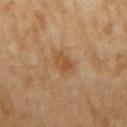Findings:
– biopsy status — imaged on a skin check; not biopsied
– image — ~15 mm crop, total-body skin-cancer survey
– patient — female, aged 68 to 72
– lesion diameter — about 2.5 mm
– location — the right upper arm
– tile lighting — cross-polarized illumination
– TBP lesion metrics — a mean CIELAB color near L≈52 a*≈21 b*≈38 and roughly 8 lightness units darker than nearby skin; a border-irregularity index near 1.5/10, a color-variation rating of about 3.5/10, and radial color variation of about 1; a lesion-detection confidence of about 100/100